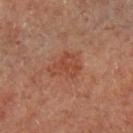Case summary:
• location · the left lower leg
• lesion diameter · ≈4 mm
• subject · male, in their mid-60s
• acquisition · ~15 mm tile from a whole-body skin photo
• lighting · cross-polarized illumination